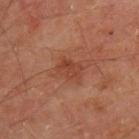patient: male, roughly 65 years of age; image: ~15 mm crop, total-body skin-cancer survey; diameter: ≈3 mm; location: the upper back.